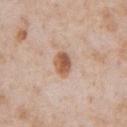The recorded lesion diameter is about 3 mm.
A male subject aged 63–67.
This image is a 15 mm lesion crop taken from a total-body photograph.
Captured under white-light illumination.
The lesion is located on the chest.